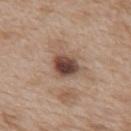Findings:
• notes · catalogued during a skin exam; not biopsied
• subject · female, roughly 40 years of age
• diameter · about 3 mm
• body site · the mid back
• imaging modality · ~15 mm tile from a whole-body skin photo
• image-analysis metrics · an area of roughly 7.5 mm², a shape eccentricity near 0.55, and two-axis asymmetry of about 0.2; roughly 16 lightness units darker than nearby skin and a normalized border contrast of about 11.5; a border-irregularity rating of about 1.5/10, internal color variation of about 6.5 on a 0–10 scale, and radial color variation of about 2; a nevus-likeness score of about 85/100 and a detector confidence of about 100 out of 100 that the crop contains a lesion